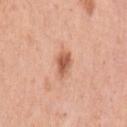Part of a total-body skin-imaging series; this lesion was reviewed on a skin check and was not flagged for biopsy. From the left upper arm. The subject is a female aged 58–62. Captured under white-light illumination. A 15 mm crop from a total-body photograph taken for skin-cancer surveillance. The lesion-visualizer software estimated border irregularity of about 2.5 on a 0–10 scale, internal color variation of about 3 on a 0–10 scale, and radial color variation of about 1.